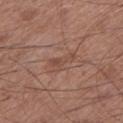Impression: Imaged during a routine full-body skin examination; the lesion was not biopsied and no histopathology is available. Context: Located on the leg. A close-up tile cropped from a whole-body skin photograph, about 15 mm across. The lesion-visualizer software estimated a lesion area of about 3.5 mm², an outline eccentricity of about 0.9 (0 = round, 1 = elongated), and two-axis asymmetry of about 0.45. The software also gave a mean CIELAB color near L≈47 a*≈21 b*≈27, about 7 CIELAB-L* units darker than the surrounding skin, and a lesion-to-skin contrast of about 5.5 (normalized; higher = more distinct). And it measured an automated nevus-likeness rating near 0 out of 100 and a detector confidence of about 100 out of 100 that the crop contains a lesion. The patient is a male aged around 60. The lesion's longest dimension is about 3.5 mm.